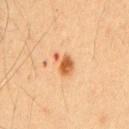Q: Is there a histopathology result?
A: catalogued during a skin exam; not biopsied
Q: What kind of image is this?
A: ~15 mm tile from a whole-body skin photo
Q: Patient demographics?
A: male, aged approximately 55
Q: What is the anatomic site?
A: the chest